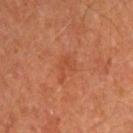Clinical impression:
Imaged during a routine full-body skin examination; the lesion was not biopsied and no histopathology is available.
Acquisition and patient details:
The patient is a male approximately 65 years of age. A 15 mm close-up extracted from a 3D total-body photography capture. Located on the right upper arm.